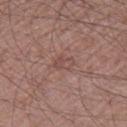Part of a total-body skin-imaging series; this lesion was reviewed on a skin check and was not flagged for biopsy.